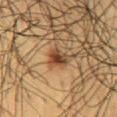Part of a total-body skin-imaging series; this lesion was reviewed on a skin check and was not flagged for biopsy. A roughly 15 mm field-of-view crop from a total-body skin photograph. A male subject roughly 60 years of age. On the front of the torso.